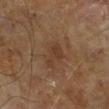<case>
<biopsy_status>not biopsied; imaged during a skin examination</biopsy_status>
<lesion_size>
  <long_diameter_mm_approx>3.0</long_diameter_mm_approx>
</lesion_size>
<patient>
  <sex>male</sex>
  <age_approx>65</age_approx>
</patient>
<image>
  <source>total-body photography crop</source>
  <field_of_view_mm>15</field_of_view_mm>
</image>
</case>